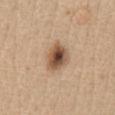Case summary:
- imaging modality: 15 mm crop, total-body photography
- subject: female, roughly 60 years of age
- location: the chest
- tile lighting: white-light illumination
- lesion diameter: ≈4 mm